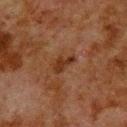Part of a total-body skin-imaging series; this lesion was reviewed on a skin check and was not flagged for biopsy. On the upper back. Approximately 3 mm at its widest. Captured under cross-polarized illumination. The subject is a male roughly 80 years of age. A 15 mm close-up tile from a total-body photography series done for melanoma screening.